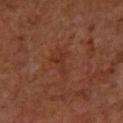Impression:
Imaged during a routine full-body skin examination; the lesion was not biopsied and no histopathology is available.
Acquisition and patient details:
A 15 mm close-up extracted from a 3D total-body photography capture. On the arm. A female subject in their mid-60s.